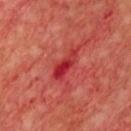Impression: Imaged during a routine full-body skin examination; the lesion was not biopsied and no histopathology is available. Context: About 4 mm across. The tile uses cross-polarized illumination. An algorithmic analysis of the crop reported a lesion color around L≈37 a*≈45 b*≈30 in CIELAB, about 10 CIELAB-L* units darker than the surrounding skin, and a normalized border contrast of about 8. And it measured a border-irregularity rating of about 4/10 and a color-variation rating of about 3/10. Located on the chest. A roughly 15 mm field-of-view crop from a total-body skin photograph. The patient is a male aged approximately 60.